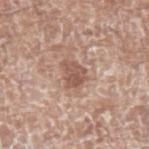No biopsy was performed on this lesion — it was imaged during a full skin examination and was not determined to be concerning.
On the left upper arm.
Automated tile analysis of the lesion measured a lesion area of about 5.5 mm² and two-axis asymmetry of about 0.4. The analysis additionally found a mean CIELAB color near L≈54 a*≈20 b*≈26, roughly 11 lightness units darker than nearby skin, and a normalized border contrast of about 7.5. The analysis additionally found border irregularity of about 4 on a 0–10 scale, a within-lesion color-variation index near 2.5/10, and peripheral color asymmetry of about 1. The software also gave a classifier nevus-likeness of about 5/100 and lesion-presence confidence of about 100/100.
This image is a 15 mm lesion crop taken from a total-body photograph.
Measured at roughly 3 mm in maximum diameter.
A male patient approximately 75 years of age.